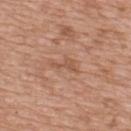The lesion was tiled from a total-body skin photograph and was not biopsied.
From the mid back.
A male patient, aged approximately 50.
Longest diameter approximately 3.5 mm.
A region of skin cropped from a whole-body photographic capture, roughly 15 mm wide.
This is a white-light tile.